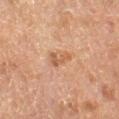Q: Was this lesion biopsied?
A: total-body-photography surveillance lesion; no biopsy
Q: Automated lesion metrics?
A: an average lesion color of about L≈49 a*≈21 b*≈32 (CIELAB), a lesion–skin lightness drop of about 8, and a normalized border contrast of about 6.5; a border-irregularity index near 6.5/10 and a color-variation rating of about 0/10; a classifier nevus-likeness of about 5/100 and a lesion-detection confidence of about 100/100
Q: Patient demographics?
A: female, aged 53 to 57
Q: What kind of image is this?
A: ~15 mm crop, total-body skin-cancer survey
Q: What lighting was used for the tile?
A: cross-polarized illumination
Q: What is the anatomic site?
A: the left thigh